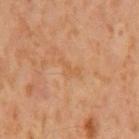Imaged during a routine full-body skin examination; the lesion was not biopsied and no histopathology is available. Measured at roughly 3 mm in maximum diameter. A male subject, roughly 60 years of age. The lesion is on the mid back. Cropped from a total-body skin-imaging series; the visible field is about 15 mm.